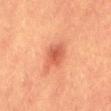Case summary:
- notes — total-body-photography surveillance lesion; no biopsy
- site — the mid back
- lesion size — about 4 mm
- image source — 15 mm crop, total-body photography
- tile lighting — cross-polarized
- subject — male, about 40 years old